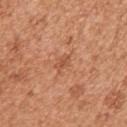  biopsy_status: not biopsied; imaged during a skin examination
  patient:
    sex: female
    age_approx: 40
  site: right upper arm
  image:
    source: total-body photography crop
    field_of_view_mm: 15